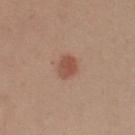Assessment: The lesion was photographed on a routine skin check and not biopsied; there is no pathology result. Background: Automated tile analysis of the lesion measured a mean CIELAB color near L≈39 a*≈18 b*≈24, roughly 8 lightness units darker than nearby skin, and a normalized lesion–skin contrast near 7. The analysis additionally found a border-irregularity index near 1.5/10, internal color variation of about 2 on a 0–10 scale, and a peripheral color-asymmetry measure near 0.5. The software also gave an automated nevus-likeness rating near 95 out of 100 and a lesion-detection confidence of about 100/100. A male subject approximately 50 years of age. Cropped from a total-body skin-imaging series; the visible field is about 15 mm. From the left forearm.